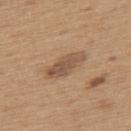The lesion was tiled from a total-body skin photograph and was not biopsied. The lesion is located on the upper back. A 15 mm close-up extracted from a 3D total-body photography capture. The subject is a male about 65 years old.